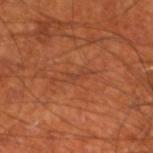  biopsy_status: not biopsied; imaged during a skin examination
  image:
    source: total-body photography crop
    field_of_view_mm: 15
  automated_metrics:
    area_mm2_approx: 2.0
    eccentricity: 0.9
    shape_asymmetry: 0.45
    border_irregularity_0_10: 6.0
    color_variation_0_10: 0.0
    peripheral_color_asymmetry: 0.0
  patient:
    sex: male
    age_approx: 70
  site: left lower leg
  lesion_size:
    long_diameter_mm_approx: 2.5